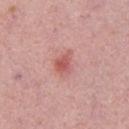{
  "lesion_size": {
    "long_diameter_mm_approx": 3.0
  },
  "patient": {
    "sex": "male",
    "age_approx": 30
  },
  "image": {
    "source": "total-body photography crop",
    "field_of_view_mm": 15
  },
  "automated_metrics": {
    "border_irregularity_0_10": 3.5,
    "color_variation_0_10": 3.5,
    "peripheral_color_asymmetry": 1.0
  }
}